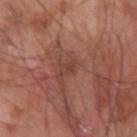  biopsy_status: not biopsied; imaged during a skin examination
  lesion_size:
    long_diameter_mm_approx: 3.0
  site: arm
  lighting: cross-polarized
  patient:
    sex: male
    age_approx: 60
  image:
    source: total-body photography crop
    field_of_view_mm: 15